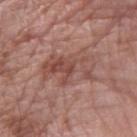The lesion was tiled from a total-body skin photograph and was not biopsied. This is a white-light tile. The lesion's longest dimension is about 6 mm. A 15 mm close-up extracted from a 3D total-body photography capture. A female subject, aged 58 to 62. On the left forearm.